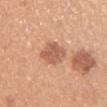{
  "biopsy_status": "not biopsied; imaged during a skin examination",
  "patient": {
    "sex": "female",
    "age_approx": 30
  },
  "lighting": "white-light",
  "site": "arm",
  "image": {
    "source": "total-body photography crop",
    "field_of_view_mm": 15
  }
}